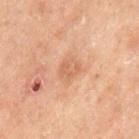| feature | finding |
|---|---|
| patient | male, aged around 70 |
| body site | the left thigh |
| tile lighting | cross-polarized |
| lesion size | ≈2.5 mm |
| automated metrics | a lesion color around L≈48 a*≈18 b*≈28 in CIELAB, a lesion–skin lightness drop of about 6, and a normalized lesion–skin contrast near 5; border irregularity of about 2 on a 0–10 scale, internal color variation of about 2 on a 0–10 scale, and a peripheral color-asymmetry measure near 1 |
| acquisition | ~15 mm tile from a whole-body skin photo |